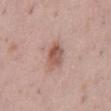follow-up = total-body-photography surveillance lesion; no biopsy | image source = ~15 mm crop, total-body skin-cancer survey | tile lighting = white-light | patient = male, aged approximately 40 | body site = the abdomen | automated lesion analysis = a border-irregularity index near 2/10, a within-lesion color-variation index near 5.5/10, and peripheral color asymmetry of about 2; an automated nevus-likeness rating near 60 out of 100 and a lesion-detection confidence of about 100/100.